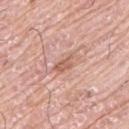Part of a total-body skin-imaging series; this lesion was reviewed on a skin check and was not flagged for biopsy.